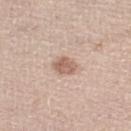Clinical impression: No biopsy was performed on this lesion — it was imaged during a full skin examination and was not determined to be concerning. Clinical summary: A male patient aged around 65. On the left lower leg. About 3 mm across. A close-up tile cropped from a whole-body skin photograph, about 15 mm across. Automated tile analysis of the lesion measured a border-irregularity rating of about 2/10, internal color variation of about 2.5 on a 0–10 scale, and radial color variation of about 1. It also reported a nevus-likeness score of about 80/100. Captured under white-light illumination.